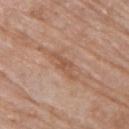From the right upper arm. The patient is a female aged approximately 75. A roughly 15 mm field-of-view crop from a total-body skin photograph.